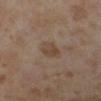{"automated_metrics": {"vs_skin_darker_L": 7.0, "vs_skin_contrast_norm": 6.5, "border_irregularity_0_10": 1.5, "peripheral_color_asymmetry": 1.0}, "lighting": "cross-polarized", "site": "left lower leg", "image": {"source": "total-body photography crop", "field_of_view_mm": 15}, "patient": {"sex": "female", "age_approx": 40}}